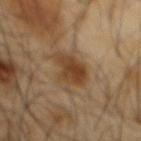Q: Was a biopsy performed?
A: imaged on a skin check; not biopsied